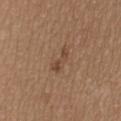Clinical impression:
Captured during whole-body skin photography for melanoma surveillance; the lesion was not biopsied.
Background:
The lesion's longest dimension is about 3 mm. A 15 mm crop from a total-body photograph taken for skin-cancer surveillance. Automated tile analysis of the lesion measured a lesion area of about 3.5 mm² and an outline eccentricity of about 0.95 (0 = round, 1 = elongated). It also reported an average lesion color of about L≈45 a*≈18 b*≈29 (CIELAB), about 8 CIELAB-L* units darker than the surrounding skin, and a normalized lesion–skin contrast near 6.5. A female patient in their 60s. Located on the head or neck. This is a white-light tile.